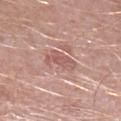follow-up=imaged on a skin check; not biopsied
subject=male, in their 50s
site=the right lower leg
lighting=white-light illumination
diameter=~4 mm (longest diameter)
acquisition=total-body-photography crop, ~15 mm field of view